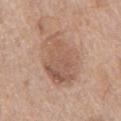  biopsy_status: not biopsied; imaged during a skin examination
  site: front of the torso
  image:
    source: total-body photography crop
    field_of_view_mm: 15
  patient:
    sex: male
    age_approx: 70
  lesion_size:
    long_diameter_mm_approx: 7.0